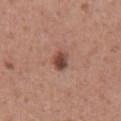Notes:
* notes · total-body-photography surveillance lesion; no biopsy
* location · the chest
* subject · male, about 45 years old
* imaging modality · 15 mm crop, total-body photography